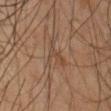  biopsy_status: not biopsied; imaged during a skin examination
  lighting: cross-polarized
  patient:
    sex: male
    age_approx: 65
  lesion_size:
    long_diameter_mm_approx: 4.0
  site: right forearm
  automated_metrics:
    area_mm2_approx: 5.0
    shape_asymmetry: 0.6
    peripheral_color_asymmetry: 0.5
    nevus_likeness_0_100: 0
    lesion_detection_confidence_0_100: 75
  image:
    source: total-body photography crop
    field_of_view_mm: 15